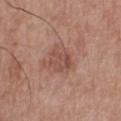{
  "image": {
    "source": "total-body photography crop",
    "field_of_view_mm": 15
  },
  "lighting": "white-light",
  "patient": {
    "sex": "male",
    "age_approx": 65
  },
  "site": "upper back"
}